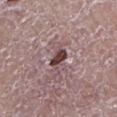Impression: The lesion was tiled from a total-body skin photograph and was not biopsied. Context: On the left lower leg. Cropped from a whole-body photographic skin survey; the tile spans about 15 mm. A male subject, roughly 50 years of age.